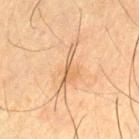workup = total-body-photography surveillance lesion; no biopsy
lesion size = about 4 mm
imaging modality = 15 mm crop, total-body photography
lighting = cross-polarized
subject = male, approximately 65 years of age
automated lesion analysis = a footprint of about 5 mm², a shape eccentricity near 0.9, and a symmetry-axis asymmetry near 0.65
location = the left thigh